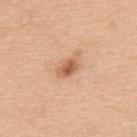Image and clinical context: The total-body-photography lesion software estimated an area of roughly 4.5 mm², an eccentricity of roughly 0.75, and two-axis asymmetry of about 0.2. And it measured a mean CIELAB color near L≈60 a*≈24 b*≈33, roughly 12 lightness units darker than nearby skin, and a lesion-to-skin contrast of about 7.5 (normalized; higher = more distinct). The analysis additionally found border irregularity of about 2 on a 0–10 scale and a peripheral color-asymmetry measure near 1. The analysis additionally found a nevus-likeness score of about 80/100. On the upper back. The subject is a male aged 48–52. The recorded lesion diameter is about 3 mm. A 15 mm close-up extracted from a 3D total-body photography capture. Captured under white-light illumination.